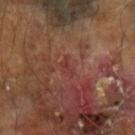Background:
The lesion is on the left forearm. This image is a 15 mm lesion crop taken from a total-body photograph. The patient is a male aged 63 to 67.